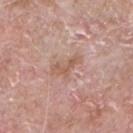No biopsy was performed on this lesion — it was imaged during a full skin examination and was not determined to be concerning. From the chest. A male patient approximately 65 years of age. This is a white-light tile. Approximately 4 mm at its widest. An algorithmic analysis of the crop reported a lesion color around L≈58 a*≈20 b*≈28 in CIELAB and a normalized border contrast of about 6. A 15 mm close-up extracted from a 3D total-body photography capture.